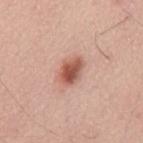No biopsy was performed on this lesion — it was imaged during a full skin examination and was not determined to be concerning.
On the mid back.
Approximately 3.5 mm at its widest.
A male subject aged 58–62.
An algorithmic analysis of the crop reported a shape eccentricity near 0.75 and a shape-asymmetry score of about 0.15 (0 = symmetric). The software also gave internal color variation of about 5 on a 0–10 scale and peripheral color asymmetry of about 1.5.
A close-up tile cropped from a whole-body skin photograph, about 15 mm across.
Imaged with white-light lighting.